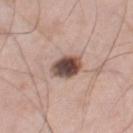notes=imaged on a skin check; not biopsied | anatomic site=the right thigh | automated metrics=an area of roughly 7.5 mm², an eccentricity of roughly 0.7, and a symmetry-axis asymmetry near 0.15; a nevus-likeness score of about 90/100 and a detector confidence of about 100 out of 100 that the crop contains a lesion | imaging modality=~15 mm tile from a whole-body skin photo | lesion diameter=about 3.5 mm | lighting=white-light | patient=male, aged around 70.